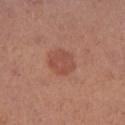biopsy status = catalogued during a skin exam; not biopsied
illumination = white-light
subject = female, roughly 40 years of age
location = the right lower leg
acquisition = ~15 mm crop, total-body skin-cancer survey
TBP lesion metrics = a border-irregularity rating of about 2/10 and radial color variation of about 0.5; a nevus-likeness score of about 65/100
diameter = ≈3.5 mm